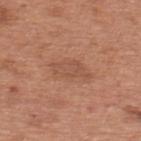Imaged during a routine full-body skin examination; the lesion was not biopsied and no histopathology is available. Cropped from a whole-body photographic skin survey; the tile spans about 15 mm. From the upper back. Automated image analysis of the tile measured a border-irregularity index near 2.5/10, internal color variation of about 2 on a 0–10 scale, and a peripheral color-asymmetry measure near 0.5. The software also gave a classifier nevus-likeness of about 0/100 and lesion-presence confidence of about 100/100. The subject is a male aged 63 to 67.